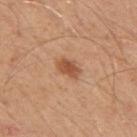The lesion was photographed on a routine skin check and not biopsied; there is no pathology result. Imaged with white-light lighting. A lesion tile, about 15 mm wide, cut from a 3D total-body photograph. The patient is a male aged approximately 50. Located on the mid back. Automated image analysis of the tile measured a lesion area of about 5 mm² and a shape eccentricity near 0.75.